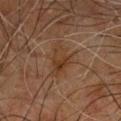Imaged during a routine full-body skin examination; the lesion was not biopsied and no histopathology is available. A male patient aged approximately 60. On the upper back. Imaged with cross-polarized lighting. Longest diameter approximately 4 mm. A 15 mm crop from a total-body photograph taken for skin-cancer surveillance.